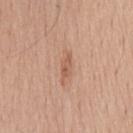  biopsy_status: not biopsied; imaged during a skin examination
  lighting: white-light
  automated_metrics:
    nevus_likeness_0_100: 10
    lesion_detection_confidence_0_100: 100
  image:
    source: total-body photography crop
    field_of_view_mm: 15
  patient:
    sex: male
    age_approx: 55